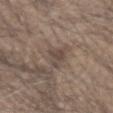No biopsy was performed on this lesion — it was imaged during a full skin examination and was not determined to be concerning. A male patient aged 68 to 72. The total-body-photography lesion software estimated internal color variation of about 2.5 on a 0–10 scale and radial color variation of about 1. The software also gave a nevus-likeness score of about 0/100 and lesion-presence confidence of about 65/100. About 3.5 mm across. This image is a 15 mm lesion crop taken from a total-body photograph. The lesion is on the head or neck.